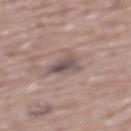Impression:
Recorded during total-body skin imaging; not selected for excision or biopsy.
Context:
From the mid back. A 15 mm crop from a total-body photograph taken for skin-cancer surveillance. A male subject, roughly 75 years of age. The tile uses white-light illumination.